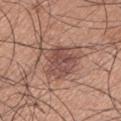Notes:
- workup · imaged on a skin check; not biopsied
- size · ≈4.5 mm
- subject · male, approximately 35 years of age
- lighting · white-light illumination
- automated lesion analysis · an area of roughly 14 mm²; a lesion color around L≈49 a*≈21 b*≈25 in CIELAB, about 10 CIELAB-L* units darker than the surrounding skin, and a normalized border contrast of about 7.5
- site · the left upper arm
- imaging modality · 15 mm crop, total-body photography